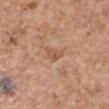The lesion was photographed on a routine skin check and not biopsied; there is no pathology result. The lesion is located on the arm. A female patient aged around 65. A 15 mm crop from a total-body photograph taken for skin-cancer surveillance. Imaged with white-light lighting. Measured at roughly 2.5 mm in maximum diameter.